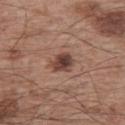Q: Is there a histopathology result?
A: imaged on a skin check; not biopsied
Q: How large is the lesion?
A: ≈2.5 mm
Q: How was this image acquired?
A: 15 mm crop, total-body photography
Q: What did automated image analysis measure?
A: a mean CIELAB color near L≈42 a*≈20 b*≈23, roughly 14 lightness units darker than nearby skin, and a normalized lesion–skin contrast near 10.5; a classifier nevus-likeness of about 70/100 and a lesion-detection confidence of about 100/100
Q: What are the patient's age and sex?
A: male, in their mid- to late 60s
Q: What is the anatomic site?
A: the left upper arm